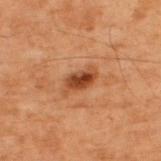<record>
  <biopsy_status>not biopsied; imaged during a skin examination</biopsy_status>
  <site>upper back</site>
  <lighting>cross-polarized</lighting>
  <patient>
    <sex>male</sex>
    <age_approx>70</age_approx>
  </patient>
  <image>
    <source>total-body photography crop</source>
    <field_of_view_mm>15</field_of_view_mm>
  </image>
  <lesion_size>
    <long_diameter_mm_approx>3.5</long_diameter_mm_approx>
  </lesion_size>
</record>